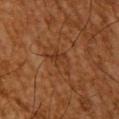The lesion was tiled from a total-body skin photograph and was not biopsied.
Measured at roughly 3.5 mm in maximum diameter.
The tile uses cross-polarized illumination.
Cropped from a whole-body photographic skin survey; the tile spans about 15 mm.
A male subject, roughly 65 years of age.
From the upper back.